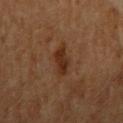A male patient aged around 60. Captured under cross-polarized illumination. Measured at roughly 4 mm in maximum diameter. Cropped from a whole-body photographic skin survey; the tile spans about 15 mm. On the left upper arm. Automated image analysis of the tile measured a lesion area of about 6.5 mm² and a shape-asymmetry score of about 0.35 (0 = symmetric). The analysis additionally found a mean CIELAB color near L≈27 a*≈18 b*≈27. The software also gave a within-lesion color-variation index near 2.5/10 and a peripheral color-asymmetry measure near 1.